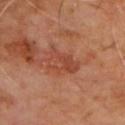Clinical impression:
Imaged during a routine full-body skin examination; the lesion was not biopsied and no histopathology is available.
Context:
Cropped from a total-body skin-imaging series; the visible field is about 15 mm. A male subject, aged 58–62. On the upper back. Measured at roughly 4 mm in maximum diameter.